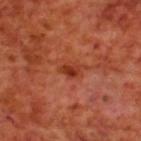follow-up: imaged on a skin check; not biopsied
body site: the upper back
automated lesion analysis: a footprint of about 4 mm², an outline eccentricity of about 0.75 (0 = round, 1 = elongated), and a shape-asymmetry score of about 0.3 (0 = symmetric); about 9 CIELAB-L* units darker than the surrounding skin; a nevus-likeness score of about 20/100 and a lesion-detection confidence of about 100/100
size: about 2.5 mm
acquisition: total-body-photography crop, ~15 mm field of view
illumination: cross-polarized
patient: male, about 70 years old